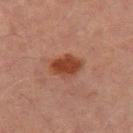automated metrics: a shape eccentricity near 0.7 and a shape-asymmetry score of about 0.15 (0 = symmetric); border irregularity of about 1.5 on a 0–10 scale and a color-variation rating of about 2/10
tile lighting: cross-polarized illumination
acquisition: 15 mm crop, total-body photography
patient: male, in their 70s
body site: the left thigh
lesion size: about 3.5 mm